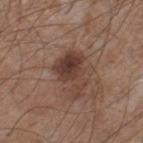On the right lower leg. A 15 mm close-up tile from a total-body photography series done for melanoma screening. Approximately 5.5 mm at its widest. Captured under white-light illumination. The patient is a male aged 53 to 57.